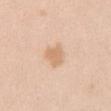Notes:
- workup: catalogued during a skin exam; not biopsied
- image source: ~15 mm crop, total-body skin-cancer survey
- TBP lesion metrics: a lesion area of about 6 mm² and an eccentricity of roughly 0.5; border irregularity of about 3 on a 0–10 scale, a color-variation rating of about 1.5/10, and peripheral color asymmetry of about 0.5; a classifier nevus-likeness of about 45/100 and lesion-presence confidence of about 100/100
- body site: the abdomen
- lesion diameter: ≈3 mm
- patient: female, in their 40s